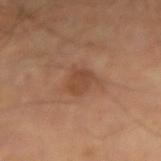Acquisition and patient details: Measured at roughly 3 mm in maximum diameter. Cropped from a whole-body photographic skin survey; the tile spans about 15 mm. Imaged with cross-polarized lighting. The subject is a male aged 58–62. The lesion is on the right forearm. Automated image analysis of the tile measured internal color variation of about 2 on a 0–10 scale and radial color variation of about 0.5. The software also gave a classifier nevus-likeness of about 5/100.